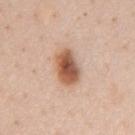<tbp_lesion>
  <biopsy_status>not biopsied; imaged during a skin examination</biopsy_status>
  <site>chest</site>
  <patient>
    <sex>male</sex>
    <age_approx>60</age_approx>
  </patient>
  <image>
    <source>total-body photography crop</source>
    <field_of_view_mm>15</field_of_view_mm>
  </image>
</tbp_lesion>